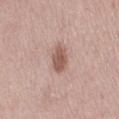Q: Was this lesion biopsied?
A: no biopsy performed (imaged during a skin exam)
Q: What is the anatomic site?
A: the right thigh
Q: What did automated image analysis measure?
A: a lesion area of about 6 mm² and an eccentricity of roughly 0.85; a lesion color around L≈55 a*≈20 b*≈25 in CIELAB, about 12 CIELAB-L* units darker than the surrounding skin, and a normalized border contrast of about 8.5; a within-lesion color-variation index near 2/10; a detector confidence of about 100 out of 100 that the crop contains a lesion
Q: Illumination type?
A: white-light illumination
Q: What is the lesion's diameter?
A: about 4 mm
Q: How was this image acquired?
A: 15 mm crop, total-body photography
Q: What are the patient's age and sex?
A: female, in their 50s